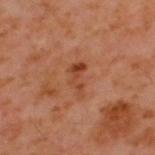biopsy_status: not biopsied; imaged during a skin examination
site: upper back
patient:
  sex: male
  age_approx: 60
image:
  source: total-body photography crop
  field_of_view_mm: 15
lesion_size:
  long_diameter_mm_approx: 4.0
lighting: cross-polarized
automated_metrics:
  cielab_L: 41
  cielab_a: 25
  cielab_b: 32
  vs_skin_darker_L: 8.0